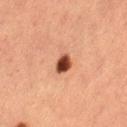Recorded during total-body skin imaging; not selected for excision or biopsy.
A close-up tile cropped from a whole-body skin photograph, about 15 mm across.
A female patient, aged 38–42.
The lesion-visualizer software estimated an area of roughly 4.5 mm², an outline eccentricity of about 0.65 (0 = round, 1 = elongated), and a symmetry-axis asymmetry near 0.2. And it measured a lesion color around L≈39 a*≈24 b*≈29 in CIELAB, roughly 18 lightness units darker than nearby skin, and a lesion-to-skin contrast of about 13.5 (normalized; higher = more distinct). And it measured a border-irregularity rating of about 1.5/10 and a color-variation rating of about 5.5/10. The analysis additionally found a classifier nevus-likeness of about 100/100 and a lesion-detection confidence of about 100/100.
The lesion is located on the left thigh.